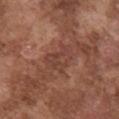Impression:
The lesion was tiled from a total-body skin photograph and was not biopsied.
Acquisition and patient details:
On the front of the torso. About 4 mm across. The lesion-visualizer software estimated an area of roughly 8 mm², a shape eccentricity near 0.75, and a shape-asymmetry score of about 0.4 (0 = symmetric). It also reported a lesion color around L≈42 a*≈22 b*≈26 in CIELAB and about 6 CIELAB-L* units darker than the surrounding skin. The software also gave a within-lesion color-variation index near 3/10. A 15 mm crop from a total-body photograph taken for skin-cancer surveillance. A male subject in their mid- to late 70s. Captured under white-light illumination.